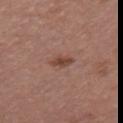Part of a total-body skin-imaging series; this lesion was reviewed on a skin check and was not flagged for biopsy. The subject is a female about 50 years old. From the front of the torso. Cropped from a whole-body photographic skin survey; the tile spans about 15 mm. An algorithmic analysis of the crop reported border irregularity of about 2.5 on a 0–10 scale, a within-lesion color-variation index near 2/10, and radial color variation of about 0.5. Captured under white-light illumination.